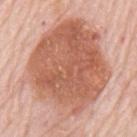{
  "biopsy_status": "not biopsied; imaged during a skin examination",
  "lesion_size": {
    "long_diameter_mm_approx": 12.0
  },
  "site": "mid back",
  "patient": {
    "sex": "male",
    "age_approx": 80
  },
  "automated_metrics": {
    "border_irregularity_0_10": 2.5,
    "color_variation_0_10": 6.0,
    "peripheral_color_asymmetry": 2.0,
    "nevus_likeness_0_100": 20,
    "lesion_detection_confidence_0_100": 100
  },
  "image": {
    "source": "total-body photography crop",
    "field_of_view_mm": 15
  },
  "lighting": "white-light"
}